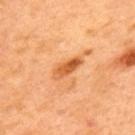The lesion was photographed on a routine skin check and not biopsied; there is no pathology result. Imaged with cross-polarized lighting. From the back. A male subject, aged around 50. Automated image analysis of the tile measured an eccentricity of roughly 0.9 and a shape-asymmetry score of about 0.2 (0 = symmetric). And it measured a border-irregularity rating of about 2.5/10 and internal color variation of about 6 on a 0–10 scale. And it measured an automated nevus-likeness rating near 40 out of 100. Cropped from a whole-body photographic skin survey; the tile spans about 15 mm. Measured at roughly 4 mm in maximum diameter.